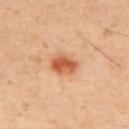Notes:
- follow-up — total-body-photography surveillance lesion; no biopsy
- patient — male, aged 48 to 52
- acquisition — ~15 mm crop, total-body skin-cancer survey
- illumination — cross-polarized
- location — the back